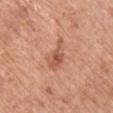The lesion was photographed on a routine skin check and not biopsied; there is no pathology result.